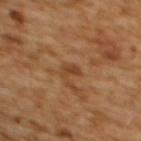No biopsy was performed on this lesion — it was imaged during a full skin examination and was not determined to be concerning. The patient is a female aged 53 to 57. This image is a 15 mm lesion crop taken from a total-body photograph. Approximately 2.5 mm at its widest. Located on the upper back. Captured under cross-polarized illumination. An algorithmic analysis of the crop reported border irregularity of about 3.5 on a 0–10 scale and a within-lesion color-variation index near 0.5/10. And it measured a lesion-detection confidence of about 100/100.